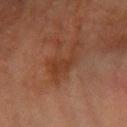Q: Was this lesion biopsied?
A: imaged on a skin check; not biopsied
Q: What is the lesion's diameter?
A: ~4.5 mm (longest diameter)
Q: Lesion location?
A: the left forearm
Q: What is the imaging modality?
A: total-body-photography crop, ~15 mm field of view
Q: Who is the patient?
A: female, aged approximately 70
Q: Automated lesion metrics?
A: a lesion area of about 7 mm² and two-axis asymmetry of about 0.5; a lesion color around L≈33 a*≈20 b*≈28 in CIELAB and about 5 CIELAB-L* units darker than the surrounding skin